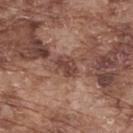The lesion was photographed on a routine skin check and not biopsied; there is no pathology result. A 15 mm crop from a total-body photograph taken for skin-cancer surveillance. The lesion is located on the upper back. A male patient, approximately 75 years of age.